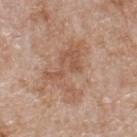The lesion was photographed on a routine skin check and not biopsied; there is no pathology result. On the upper back. A male subject in their 80s. An algorithmic analysis of the crop reported an average lesion color of about L≈55 a*≈20 b*≈30 (CIELAB), a lesion–skin lightness drop of about 8, and a lesion-to-skin contrast of about 6 (normalized; higher = more distinct). The software also gave border irregularity of about 10 on a 0–10 scale and radial color variation of about 1. A 15 mm crop from a total-body photograph taken for skin-cancer surveillance. Approximately 7 mm at its widest.